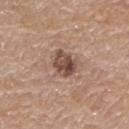This image is a 15 mm lesion crop taken from a total-body photograph.
Located on the left upper arm.
The lesion-visualizer software estimated a footprint of about 7 mm², an eccentricity of roughly 0.6, and a symmetry-axis asymmetry near 0.25. The analysis additionally found a mean CIELAB color near L≈48 a*≈18 b*≈24, a lesion–skin lightness drop of about 14, and a lesion-to-skin contrast of about 10 (normalized; higher = more distinct). It also reported a border-irregularity rating of about 2.5/10.
A male patient, aged approximately 80.
The tile uses white-light illumination.